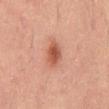No biopsy was performed on this lesion — it was imaged during a full skin examination and was not determined to be concerning. The subject is a male aged 53 to 57. The tile uses cross-polarized illumination. The lesion is on the abdomen. Cropped from a whole-body photographic skin survey; the tile spans about 15 mm.